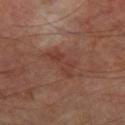This lesion was catalogued during total-body skin photography and was not selected for biopsy. A close-up tile cropped from a whole-body skin photograph, about 15 mm across. Longest diameter approximately 4.5 mm. A male subject, aged 68 to 72. Automated tile analysis of the lesion measured a lesion–skin lightness drop of about 6 and a normalized border contrast of about 5.5. The software also gave a color-variation rating of about 3/10 and peripheral color asymmetry of about 1. The lesion is located on the right thigh. This is a cross-polarized tile.